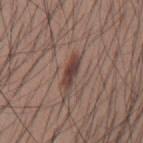follow-up = total-body-photography surveillance lesion; no biopsy
lighting = white-light illumination
site = the mid back
imaging modality = ~15 mm tile from a whole-body skin photo
subject = male, aged 33 to 37